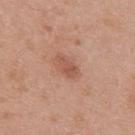acquisition = 15 mm crop, total-body photography | patient = female, about 40 years old | anatomic site = the upper back | image-analysis metrics = an area of roughly 4.5 mm²; a lesion color around L≈54 a*≈23 b*≈30 in CIELAB and a normalized lesion–skin contrast near 6; border irregularity of about 2.5 on a 0–10 scale, a color-variation rating of about 2.5/10, and peripheral color asymmetry of about 1 | lighting = white-light | lesion size = ≈3 mm.